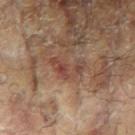Assessment:
Imaged during a routine full-body skin examination; the lesion was not biopsied and no histopathology is available.
Background:
A female subject aged around 80. An algorithmic analysis of the crop reported a lesion area of about 6.5 mm² and a shape-asymmetry score of about 0.6 (0 = symmetric). The analysis additionally found roughly 7 lightness units darker than nearby skin and a normalized border contrast of about 6. This is a cross-polarized tile. The lesion is located on the right arm. A 15 mm close-up extracted from a 3D total-body photography capture.